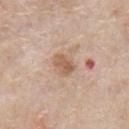workup: no biopsy performed (imaged during a skin exam)
size: ~3 mm (longest diameter)
patient: male, approximately 65 years of age
anatomic site: the chest
imaging modality: ~15 mm tile from a whole-body skin photo